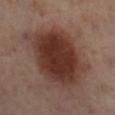Captured during whole-body skin photography for melanoma surveillance; the lesion was not biopsied. A 15 mm close-up tile from a total-body photography series done for melanoma screening. The tile uses cross-polarized illumination. From the right lower leg. An algorithmic analysis of the crop reported an area of roughly 39 mm², a shape eccentricity near 0.65, and a symmetry-axis asymmetry near 0.15. It also reported a lesion color around L≈35 a*≈21 b*≈24 in CIELAB and a lesion-to-skin contrast of about 11.5 (normalized; higher = more distinct). It also reported an automated nevus-likeness rating near 100 out of 100 and a detector confidence of about 100 out of 100 that the crop contains a lesion. The lesion's longest dimension is about 8.5 mm. A female patient in their mid- to late 50s.